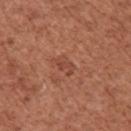biopsy status: total-body-photography surveillance lesion; no biopsy
subject: female, about 50 years old
image-analysis metrics: a border-irregularity index near 2.5/10, a color-variation rating of about 2/10, and radial color variation of about 1
location: the right upper arm
diameter: ~2.5 mm (longest diameter)
image: ~15 mm crop, total-body skin-cancer survey